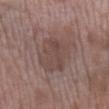Clinical impression:
Captured during whole-body skin photography for melanoma surveillance; the lesion was not biopsied.
Image and clinical context:
A female patient, aged approximately 70. The tile uses white-light illumination. From the leg. The lesion's longest dimension is about 5.5 mm. A lesion tile, about 15 mm wide, cut from a 3D total-body photograph. The total-body-photography lesion software estimated about 6 CIELAB-L* units darker than the surrounding skin and a normalized lesion–skin contrast near 5.5.